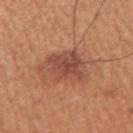notes: imaged on a skin check; not biopsied | lighting: white-light | lesion diameter: ~4.5 mm (longest diameter) | image: ~15 mm tile from a whole-body skin photo | body site: the right upper arm | patient: male, roughly 55 years of age | automated lesion analysis: an average lesion color of about L≈48 a*≈24 b*≈30 (CIELAB), about 9 CIELAB-L* units darker than the surrounding skin, and a normalized border contrast of about 7.5; a color-variation rating of about 4.5/10 and a peripheral color-asymmetry measure near 1.5; an automated nevus-likeness rating near 20 out of 100 and a detector confidence of about 100 out of 100 that the crop contains a lesion.